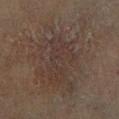biopsy status: no biopsy performed (imaged during a skin exam)
imaging modality: total-body-photography crop, ~15 mm field of view
size: about 6 mm
location: the leg
patient: male, approximately 70 years of age